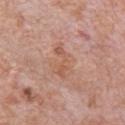Captured during whole-body skin photography for melanoma surveillance; the lesion was not biopsied. The recorded lesion diameter is about 4.5 mm. This image is a 15 mm lesion crop taken from a total-body photograph. Located on the chest. Captured under white-light illumination. A male patient in their 80s.